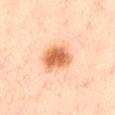Recorded during total-body skin imaging; not selected for excision or biopsy. The tile uses cross-polarized illumination. A 15 mm close-up extracted from a 3D total-body photography capture. Located on the abdomen. Longest diameter approximately 4 mm. A male subject aged around 85 or older.